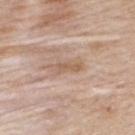Q: Lesion location?
A: the back
Q: What lighting was used for the tile?
A: white-light illumination
Q: How was this image acquired?
A: 15 mm crop, total-body photography
Q: Who is the patient?
A: female, aged 43 to 47
Q: Automated lesion metrics?
A: a border-irregularity index near 4/10, a within-lesion color-variation index near 1/10, and radial color variation of about 0; a nevus-likeness score of about 0/100 and a lesion-detection confidence of about 70/100
Q: How large is the lesion?
A: ≈3.5 mm